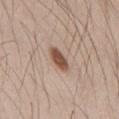workup = total-body-photography surveillance lesion; no biopsy.